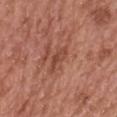{"biopsy_status": "not biopsied; imaged during a skin examination", "site": "upper back", "lighting": "white-light", "patient": {"sex": "female", "age_approx": 60}, "image": {"source": "total-body photography crop", "field_of_view_mm": 15}}